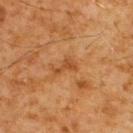Q: Is there a histopathology result?
A: imaged on a skin check; not biopsied
Q: What is the anatomic site?
A: the upper back
Q: Who is the patient?
A: male, roughly 60 years of age
Q: What kind of image is this?
A: ~15 mm tile from a whole-body skin photo